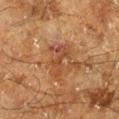Impression: Imaged during a routine full-body skin examination; the lesion was not biopsied and no histopathology is available. Image and clinical context: A male subject, aged 58–62. Located on the right lower leg. A 15 mm close-up extracted from a 3D total-body photography capture. The tile uses cross-polarized illumination. The lesion's longest dimension is about 4 mm.